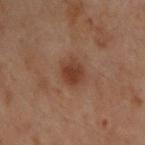Acquisition and patient details: The patient is a male aged around 30. A 15 mm close-up tile from a total-body photography series done for melanoma screening. The lesion is located on the back.